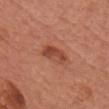Clinical impression: Imaged during a routine full-body skin examination; the lesion was not biopsied and no histopathology is available. Image and clinical context: Measured at roughly 3.5 mm in maximum diameter. This image is a 15 mm lesion crop taken from a total-body photograph. Located on the front of the torso. Automated tile analysis of the lesion measured a footprint of about 6 mm², an outline eccentricity of about 0.85 (0 = round, 1 = elongated), and a shape-asymmetry score of about 0.25 (0 = symmetric). And it measured an automated nevus-likeness rating near 55 out of 100 and a lesion-detection confidence of about 100/100. The tile uses white-light illumination. A female subject approximately 65 years of age.